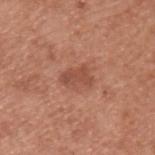| key | value |
|---|---|
| biopsy status | catalogued during a skin exam; not biopsied |
| automated metrics | an area of roughly 5 mm² and a shape eccentricity near 0.8; a lesion–skin lightness drop of about 9 and a normalized border contrast of about 6.5; a classifier nevus-likeness of about 20/100 |
| lesion size | about 3.5 mm |
| anatomic site | the upper back |
| patient | male, aged approximately 55 |
| image source | total-body-photography crop, ~15 mm field of view |